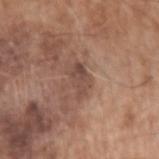follow-up: catalogued during a skin exam; not biopsied.